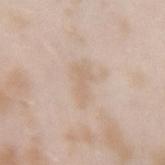{
  "biopsy_status": "not biopsied; imaged during a skin examination",
  "lighting": "white-light",
  "site": "left forearm",
  "image": {
    "source": "total-body photography crop",
    "field_of_view_mm": 15
  },
  "lesion_size": {
    "long_diameter_mm_approx": 4.5
  },
  "patient": {
    "sex": "female",
    "age_approx": 25
  }
}